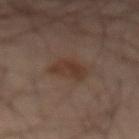No biopsy was performed on this lesion — it was imaged during a full skin examination and was not determined to be concerning.
A male subject roughly 70 years of age.
Measured at roughly 3.5 mm in maximum diameter.
An algorithmic analysis of the crop reported a footprint of about 8.5 mm² and two-axis asymmetry of about 0.25. The analysis additionally found lesion-presence confidence of about 100/100.
A 15 mm close-up tile from a total-body photography series done for melanoma screening.
Located on the front of the torso.